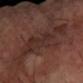biopsy status: imaged on a skin check; not biopsied
subject: male, aged around 65
lighting: cross-polarized illumination
location: the right forearm
acquisition: ~15 mm crop, total-body skin-cancer survey
size: about 6.5 mm